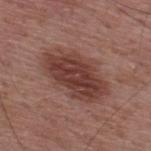Imaged during a routine full-body skin examination; the lesion was not biopsied and no histopathology is available. A male patient, aged 53–57. This is a white-light tile. On the upper back. The lesion's longest dimension is about 7.5 mm. A close-up tile cropped from a whole-body skin photograph, about 15 mm across. Automated tile analysis of the lesion measured a shape eccentricity near 0.85. It also reported a mean CIELAB color near L≈40 a*≈22 b*≈23, roughly 11 lightness units darker than nearby skin, and a normalized lesion–skin contrast near 9.